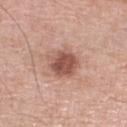{"biopsy_status": "not biopsied; imaged during a skin examination", "lighting": "white-light", "patient": {"sex": "male", "age_approx": 80}, "site": "right lower leg", "image": {"source": "total-body photography crop", "field_of_view_mm": 15}, "lesion_size": {"long_diameter_mm_approx": 3.5}}